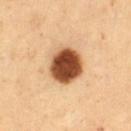| field | value |
|---|---|
| follow-up | total-body-photography surveillance lesion; no biopsy |
| size | about 4.5 mm |
| tile lighting | cross-polarized |
| subject | female, approximately 50 years of age |
| body site | the right thigh |
| acquisition | ~15 mm tile from a whole-body skin photo |